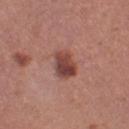{
  "biopsy_status": "not biopsied; imaged during a skin examination",
  "patient": {
    "sex": "female",
    "age_approx": 30
  },
  "lesion_size": {
    "long_diameter_mm_approx": 3.5
  },
  "site": "left thigh",
  "image": {
    "source": "total-body photography crop",
    "field_of_view_mm": 15
  }
}